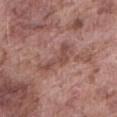Part of a total-body skin-imaging series; this lesion was reviewed on a skin check and was not flagged for biopsy.
Imaged with white-light lighting.
A region of skin cropped from a whole-body photographic capture, roughly 15 mm wide.
The recorded lesion diameter is about 5.5 mm.
From the abdomen.
A male patient about 75 years old.